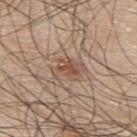Impression: Recorded during total-body skin imaging; not selected for excision or biopsy. Background: The tile uses white-light illumination. A male patient aged around 45. The recorded lesion diameter is about 4 mm. Located on the upper back. A 15 mm close-up tile from a total-body photography series done for melanoma screening. Automated tile analysis of the lesion measured roughly 9 lightness units darker than nearby skin and a normalized lesion–skin contrast near 6.5. The software also gave a border-irregularity index near 4/10 and a peripheral color-asymmetry measure near 1.